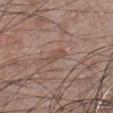Findings:
* biopsy status — catalogued during a skin exam; not biopsied
* size — ≈3 mm
* imaging modality — 15 mm crop, total-body photography
* lighting — white-light illumination
* subject — male, approximately 70 years of age
* anatomic site — the chest